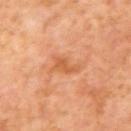Q: Is there a histopathology result?
A: catalogued during a skin exam; not biopsied
Q: What are the patient's age and sex?
A: male, aged 58 to 62
Q: Lesion location?
A: the upper back
Q: What is the lesion's diameter?
A: ~3.5 mm (longest diameter)
Q: How was this image acquired?
A: ~15 mm tile from a whole-body skin photo